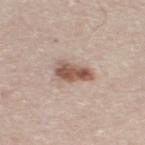tile lighting: white-light | anatomic site: the left thigh | image-analysis metrics: a lesion color around L≈55 a*≈18 b*≈26 in CIELAB, a lesion–skin lightness drop of about 14, and a normalized lesion–skin contrast near 9.5; border irregularity of about 3 on a 0–10 scale, a within-lesion color-variation index near 5/10, and radial color variation of about 1.5; lesion-presence confidence of about 100/100 | acquisition: ~15 mm tile from a whole-body skin photo | subject: male, aged approximately 70.